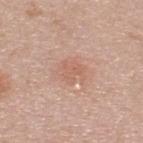Case summary:
• notes: catalogued during a skin exam; not biopsied
• tile lighting: white-light
• size: ≈3 mm
• imaging modality: 15 mm crop, total-body photography
• anatomic site: the back
• subject: male, roughly 45 years of age